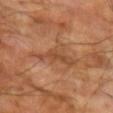No biopsy was performed on this lesion — it was imaged during a full skin examination and was not determined to be concerning. From the left upper arm. Cropped from a total-body skin-imaging series; the visible field is about 15 mm. The patient is a male about 70 years old.